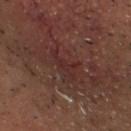No biopsy was performed on this lesion — it was imaged during a full skin examination and was not determined to be concerning. A male subject, aged approximately 60. Located on the head or neck. A region of skin cropped from a whole-body photographic capture, roughly 15 mm wide. Longest diameter approximately 4 mm.